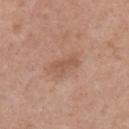Clinical impression: Part of a total-body skin-imaging series; this lesion was reviewed on a skin check and was not flagged for biopsy. Background: A roughly 15 mm field-of-view crop from a total-body skin photograph. A female subject, about 40 years old. Located on the left upper arm.